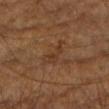{"image": {"source": "total-body photography crop", "field_of_view_mm": 15}, "lighting": "cross-polarized", "lesion_size": {"long_diameter_mm_approx": 4.0}, "site": "right upper arm", "patient": {"sex": "male", "age_approx": "85+"}}